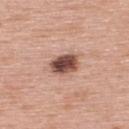Case summary:
• notes — total-body-photography surveillance lesion; no biopsy
• illumination — white-light illumination
• imaging modality — ~15 mm crop, total-body skin-cancer survey
• automated lesion analysis — a lesion color around L≈50 a*≈22 b*≈25 in CIELAB and roughly 19 lightness units darker than nearby skin; a border-irregularity rating of about 1.5/10, a color-variation rating of about 6.5/10, and peripheral color asymmetry of about 2; a nevus-likeness score of about 90/100 and lesion-presence confidence of about 100/100
• size — ~3.5 mm (longest diameter)
• patient — male, in their 60s
• site — the upper back